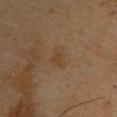The lesion was tiled from a total-body skin photograph and was not biopsied. The lesion is located on the upper back. Longest diameter approximately 2.5 mm. A male subject aged 68 to 72. Cropped from a total-body skin-imaging series; the visible field is about 15 mm.